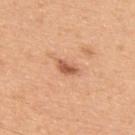No biopsy was performed on this lesion — it was imaged during a full skin examination and was not determined to be concerning. A male patient, aged around 50. Measured at roughly 3 mm in maximum diameter. Imaged with white-light lighting. The lesion-visualizer software estimated a lesion area of about 3.5 mm², an outline eccentricity of about 0.85 (0 = round, 1 = elongated), and a symmetry-axis asymmetry near 0.25. It also reported about 12 CIELAB-L* units darker than the surrounding skin. The software also gave an automated nevus-likeness rating near 50 out of 100 and a detector confidence of about 100 out of 100 that the crop contains a lesion. A 15 mm crop from a total-body photograph taken for skin-cancer surveillance. The lesion is on the upper back.